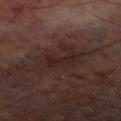  biopsy_status: not biopsied; imaged during a skin examination
  image:
    source: total-body photography crop
    field_of_view_mm: 15
  lesion_size:
    long_diameter_mm_approx: 6.0
  site: leg
  patient:
    sex: male
    age_approx: 70
  lighting: cross-polarized
  automated_metrics:
    border_irregularity_0_10: 6.5
    color_variation_0_10: 3.0
    peripheral_color_asymmetry: 1.0